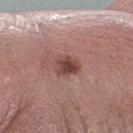Q: Was this lesion biopsied?
A: catalogued during a skin exam; not biopsied
Q: What is the anatomic site?
A: the right forearm
Q: What lighting was used for the tile?
A: white-light illumination
Q: What is the imaging modality?
A: total-body-photography crop, ~15 mm field of view
Q: Who is the patient?
A: male, aged approximately 65
Q: What did automated image analysis measure?
A: a lesion area of about 6 mm² and an eccentricity of roughly 0.55; a lesion–skin lightness drop of about 12 and a lesion-to-skin contrast of about 9 (normalized; higher = more distinct); an automated nevus-likeness rating near 75 out of 100 and lesion-presence confidence of about 100/100
Q: What is the lesion's diameter?
A: ≈3 mm